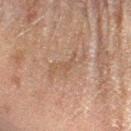Assessment:
Part of a total-body skin-imaging series; this lesion was reviewed on a skin check and was not flagged for biopsy.
Clinical summary:
This image is a 15 mm lesion crop taken from a total-body photograph. Measured at roughly 5 mm in maximum diameter. This is a cross-polarized tile. The patient is a male aged approximately 60. On the left forearm.